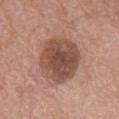workup: imaged on a skin check; not biopsied
size: ~5.5 mm (longest diameter)
TBP lesion metrics: a footprint of about 23 mm², a shape eccentricity near 0.45, and a shape-asymmetry score of about 0.1 (0 = symmetric); a lesion color around L≈49 a*≈22 b*≈27 in CIELAB, a lesion–skin lightness drop of about 13, and a normalized lesion–skin contrast near 9; a nevus-likeness score of about 10/100
subject: female, approximately 65 years of age
acquisition: ~15 mm crop, total-body skin-cancer survey
location: the upper back